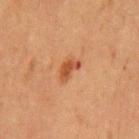biopsy status: total-body-photography surveillance lesion; no biopsy | image-analysis metrics: a classifier nevus-likeness of about 90/100 and a lesion-detection confidence of about 100/100 | body site: the chest | diameter: ≈3 mm | imaging modality: 15 mm crop, total-body photography | subject: male, aged around 65 | lighting: cross-polarized illumination.